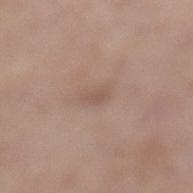notes: imaged on a skin check; not biopsied | image source: 15 mm crop, total-body photography | subject: female, roughly 60 years of age | size: about 2.5 mm | body site: the left lower leg.